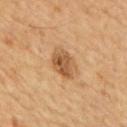Impression: Imaged during a routine full-body skin examination; the lesion was not biopsied and no histopathology is available. Background: Captured under cross-polarized illumination. The total-body-photography lesion software estimated an area of roughly 8 mm², an outline eccentricity of about 0.75 (0 = round, 1 = elongated), and a symmetry-axis asymmetry near 0.15. The analysis additionally found a lesion color around L≈52 a*≈19 b*≈36 in CIELAB, about 11 CIELAB-L* units darker than the surrounding skin, and a normalized border contrast of about 8. A 15 mm close-up extracted from a 3D total-body photography capture. The recorded lesion diameter is about 4 mm. The patient is a male aged 63 to 67. From the upper back.